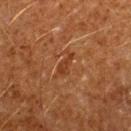workup=imaged on a skin check; not biopsied
automated metrics=a border-irregularity rating of about 4/10, a within-lesion color-variation index near 0.5/10, and peripheral color asymmetry of about 0; a classifier nevus-likeness of about 0/100
patient=male, in their 60s
image=~15 mm crop, total-body skin-cancer survey
anatomic site=the left forearm
size=≈3 mm